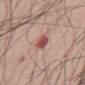notes: catalogued during a skin exam; not biopsied | acquisition: 15 mm crop, total-body photography | anatomic site: the right upper arm | subject: male, in their 40s.